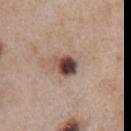Clinical impression: Imaged during a routine full-body skin examination; the lesion was not biopsied and no histopathology is available. Background: A 15 mm close-up tile from a total-body photography series done for melanoma screening. Located on the front of the torso. A female subject in their 40s. The lesion-visualizer software estimated an area of roughly 6 mm² and a symmetry-axis asymmetry near 0.2. The analysis additionally found a lesion color around L≈44 a*≈17 b*≈22 in CIELAB, about 19 CIELAB-L* units darker than the surrounding skin, and a lesion-to-skin contrast of about 13.5 (normalized; higher = more distinct). And it measured a within-lesion color-variation index near 10/10 and a peripheral color-asymmetry measure near 4.